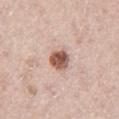Findings:
• workup: catalogued during a skin exam; not biopsied
• acquisition: ~15 mm tile from a whole-body skin photo
• tile lighting: white-light illumination
• patient: male, aged 63–67
• diameter: about 3 mm
• site: the left thigh